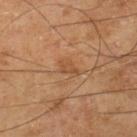Captured during whole-body skin photography for melanoma surveillance; the lesion was not biopsied. The tile uses cross-polarized illumination. A lesion tile, about 15 mm wide, cut from a 3D total-body photograph. The lesion's longest dimension is about 2.5 mm. A male subject in their mid- to late 60s. The lesion is located on the right lower leg.